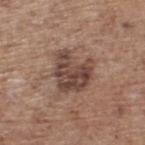  patient:
    sex: male
    age_approx: 70
  lighting: white-light
  site: upper back
  image:
    source: total-body photography crop
    field_of_view_mm: 15
  lesion_size:
    long_diameter_mm_approx: 5.0